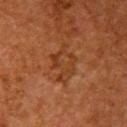Impression:
No biopsy was performed on this lesion — it was imaged during a full skin examination and was not determined to be concerning.
Context:
A female patient in their 50s. The lesion is located on the right upper arm. A 15 mm crop from a total-body photograph taken for skin-cancer surveillance.